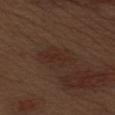Captured during whole-body skin photography for melanoma surveillance; the lesion was not biopsied. A close-up tile cropped from a whole-body skin photograph, about 15 mm across. Imaged with white-light lighting. About 5 mm across. The lesion is located on the left upper arm. A male patient roughly 70 years of age. An algorithmic analysis of the crop reported an average lesion color of about L≈27 a*≈18 b*≈22 (CIELAB), roughly 5 lightness units darker than nearby skin, and a normalized border contrast of about 5.5. The software also gave border irregularity of about 2.5 on a 0–10 scale, a within-lesion color-variation index near 2.5/10, and a peripheral color-asymmetry measure near 1. The software also gave an automated nevus-likeness rating near 30 out of 100.